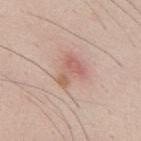workup: total-body-photography surveillance lesion; no biopsy
automated lesion analysis: two-axis asymmetry of about 0.6; border irregularity of about 6.5 on a 0–10 scale, a color-variation rating of about 3/10, and radial color variation of about 0.5; a nevus-likeness score of about 50/100 and a detector confidence of about 100 out of 100 that the crop contains a lesion
image: ~15 mm tile from a whole-body skin photo
size: ~3.5 mm (longest diameter)
subject: male, aged approximately 35
site: the mid back
lighting: white-light illumination